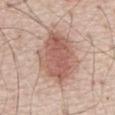{
  "biopsy_status": "not biopsied; imaged during a skin examination",
  "patient": {
    "sex": "male",
    "age_approx": 70
  },
  "lighting": "white-light",
  "image": {
    "source": "total-body photography crop",
    "field_of_view_mm": 15
  },
  "automated_metrics": {
    "cielab_L": 58,
    "cielab_a": 21,
    "cielab_b": 26,
    "vs_skin_darker_L": 12.0,
    "vs_skin_contrast_norm": 8.0,
    "color_variation_0_10": 4.5,
    "peripheral_color_asymmetry": 1.5,
    "lesion_detection_confidence_0_100": 100
  },
  "lesion_size": {
    "long_diameter_mm_approx": 7.5
  },
  "site": "chest"
}